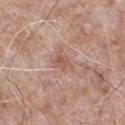Impression:
Recorded during total-body skin imaging; not selected for excision or biopsy.
Clinical summary:
On the left lower leg. Cropped from a whole-body photographic skin survey; the tile spans about 15 mm. About 2.5 mm across. The patient is a male aged approximately 75. Automated image analysis of the tile measured a lesion area of about 3.5 mm², an eccentricity of roughly 0.7, and a shape-asymmetry score of about 0.3 (0 = symmetric). It also reported a mean CIELAB color near L≈55 a*≈22 b*≈28 and a lesion–skin lightness drop of about 7. And it measured a nevus-likeness score of about 0/100 and a detector confidence of about 100 out of 100 that the crop contains a lesion.